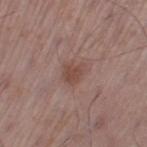This lesion was catalogued during total-body skin photography and was not selected for biopsy.
The recorded lesion diameter is about 2.5 mm.
A lesion tile, about 15 mm wide, cut from a 3D total-body photograph.
The lesion is located on the leg.
A male patient in their mid-50s.
The tile uses white-light illumination.
An algorithmic analysis of the crop reported a border-irregularity rating of about 3/10 and a peripheral color-asymmetry measure near 0.5. The analysis additionally found a nevus-likeness score of about 45/100 and lesion-presence confidence of about 100/100.